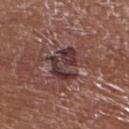Notes:
• follow-up · imaged on a skin check; not biopsied
• patient · male, in their mid- to late 70s
• image-analysis metrics · a lesion area of about 9 mm², a shape eccentricity near 0.75, and a shape-asymmetry score of about 0.45 (0 = symmetric); a within-lesion color-variation index near 4.5/10 and radial color variation of about 1.5; an automated nevus-likeness rating near 0 out of 100 and a lesion-detection confidence of about 100/100
• imaging modality · ~15 mm crop, total-body skin-cancer survey
• tile lighting · white-light illumination
• size · ≈4.5 mm
• body site · the left lower leg